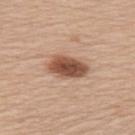Findings:
- biopsy status · no biopsy performed (imaged during a skin exam)
- lighting · white-light
- body site · the upper back
- patient · female, roughly 60 years of age
- size · about 4.5 mm
- image source · ~15 mm tile from a whole-body skin photo